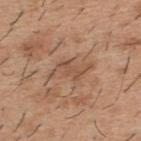Q: Is there a histopathology result?
A: imaged on a skin check; not biopsied
Q: What is the imaging modality?
A: 15 mm crop, total-body photography
Q: Illumination type?
A: white-light
Q: What is the anatomic site?
A: the upper back
Q: Automated lesion metrics?
A: a shape eccentricity near 0.9 and two-axis asymmetry of about 0.35; an average lesion color of about L≈51 a*≈20 b*≈31 (CIELAB), about 7 CIELAB-L* units darker than the surrounding skin, and a normalized border contrast of about 5
Q: Lesion size?
A: ~3 mm (longest diameter)
Q: Patient demographics?
A: male, aged 38 to 42